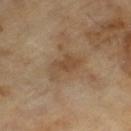The lesion was tiled from a total-body skin photograph and was not biopsied. Measured at roughly 4.5 mm in maximum diameter. A male subject approximately 65 years of age. A close-up tile cropped from a whole-body skin photograph, about 15 mm across.